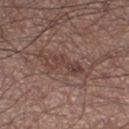Context: About 4.5 mm across. A male subject aged 53–57. The lesion-visualizer software estimated an area of roughly 7 mm², an outline eccentricity of about 0.9 (0 = round, 1 = elongated), and two-axis asymmetry of about 0.45. The software also gave a lesion color around L≈41 a*≈18 b*≈22 in CIELAB and a normalized border contrast of about 6. The analysis additionally found a border-irregularity index near 5.5/10 and internal color variation of about 5.5 on a 0–10 scale. It also reported an automated nevus-likeness rating near 5 out of 100 and lesion-presence confidence of about 95/100. Located on the right thigh. This image is a 15 mm lesion crop taken from a total-body photograph.